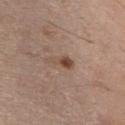Findings:
• biopsy status: total-body-photography surveillance lesion; no biopsy
• image-analysis metrics: a footprint of about 4 mm², an eccentricity of roughly 0.9, and a symmetry-axis asymmetry near 0.55; an average lesion color of about L≈48 a*≈17 b*≈26 (CIELAB), roughly 10 lightness units darker than nearby skin, and a normalized lesion–skin contrast near 7.5; a border-irregularity index near 5.5/10, a within-lesion color-variation index near 2.5/10, and a peripheral color-asymmetry measure near 1
• subject: male, about 60 years old
• lighting: white-light
• acquisition: 15 mm crop, total-body photography
• anatomic site: the chest